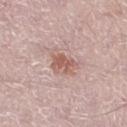Assessment:
Recorded during total-body skin imaging; not selected for excision or biopsy.
Clinical summary:
On the left lower leg. A male subject, aged 48 to 52. A 15 mm close-up tile from a total-body photography series done for melanoma screening.